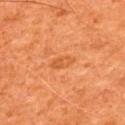Captured during whole-body skin photography for melanoma surveillance; the lesion was not biopsied.